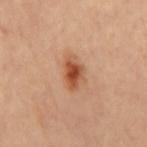Clinical impression:
The lesion was photographed on a routine skin check and not biopsied; there is no pathology result.
Clinical summary:
From the mid back. A 15 mm close-up extracted from a 3D total-body photography capture.